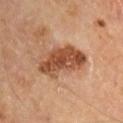notes: catalogued during a skin exam; not biopsied
lesion size: ≈6.5 mm
body site: the arm
patient: male, approximately 65 years of age
image: 15 mm crop, total-body photography